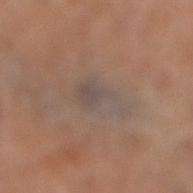The lesion was tiled from a total-body skin photograph and was not biopsied.
A female subject, about 65 years old.
The lesion's longest dimension is about 5.5 mm.
A 15 mm crop from a total-body photograph taken for skin-cancer surveillance.
The tile uses white-light illumination.
From the left leg.